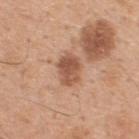workup: no biopsy performed (imaged during a skin exam) | patient: male, aged around 50 | automated lesion analysis: a mean CIELAB color near L≈54 a*≈23 b*≈31, about 12 CIELAB-L* units darker than the surrounding skin, and a lesion-to-skin contrast of about 8 (normalized; higher = more distinct); a nevus-likeness score of about 25/100 and a detector confidence of about 100 out of 100 that the crop contains a lesion | lighting: white-light illumination | anatomic site: the back | image: total-body-photography crop, ~15 mm field of view | diameter: ≈3.5 mm.